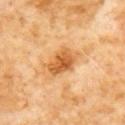Acquisition and patient details: The lesion is on the chest. Approximately 4 mm at its widest. The patient is a male about 60 years old. This is a cross-polarized tile. This image is a 15 mm lesion crop taken from a total-body photograph. An algorithmic analysis of the crop reported a symmetry-axis asymmetry near 0.25. And it measured an average lesion color of about L≈58 a*≈25 b*≈45 (CIELAB) and about 12 CIELAB-L* units darker than the surrounding skin. The analysis additionally found a border-irregularity index near 2.5/10 and a within-lesion color-variation index near 4.5/10.